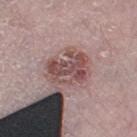  biopsy_status: not biopsied; imaged during a skin examination
  lesion_size:
    long_diameter_mm_approx: 5.0
  automated_metrics:
    area_mm2_approx: 14.0
    eccentricity: 0.7
    shape_asymmetry: 0.25
    cielab_L: 49
    cielab_a: 20
    cielab_b: 19
    vs_skin_darker_L: 12.0
    vs_skin_contrast_norm: 8.5
    border_irregularity_0_10: 4.0
    color_variation_0_10: 6.0
    peripheral_color_asymmetry: 2.0
  lighting: white-light
  site: left thigh
  patient:
    sex: female
    age_approx: 65
  image:
    source: total-body photography crop
    field_of_view_mm: 15